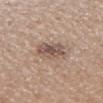biopsy status = imaged on a skin check; not biopsied
lighting = white-light illumination
body site = the right upper arm
imaging modality = 15 mm crop, total-body photography
subject = male, roughly 55 years of age